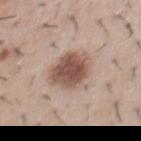No biopsy was performed on this lesion — it was imaged during a full skin examination and was not determined to be concerning. The total-body-photography lesion software estimated a border-irregularity rating of about 2/10, a color-variation rating of about 4.5/10, and radial color variation of about 1. The analysis additionally found an automated nevus-likeness rating near 95 out of 100. A male patient roughly 30 years of age. This image is a 15 mm lesion crop taken from a total-body photograph. Longest diameter approximately 4.5 mm. The tile uses white-light illumination. The lesion is located on the chest.